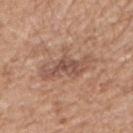Captured during whole-body skin photography for melanoma surveillance; the lesion was not biopsied.
The lesion's longest dimension is about 5.5 mm.
A 15 mm close-up extracted from a 3D total-body photography capture.
This is a white-light tile.
The subject is a male about 60 years old.
The lesion is on the right upper arm.